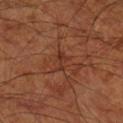<lesion>
<site>right lower leg</site>
<lighting>cross-polarized</lighting>
<lesion_size>
  <long_diameter_mm_approx>2.5</long_diameter_mm_approx>
</lesion_size>
<patient>
  <age_approx>65</age_approx>
</patient>
<image>
  <source>total-body photography crop</source>
  <field_of_view_mm>15</field_of_view_mm>
</image>
</lesion>